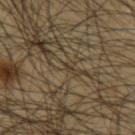| field | value |
|---|---|
| biopsy status | imaged on a skin check; not biopsied |
| lesion size | ≈1 mm |
| patient | male, roughly 45 years of age |
| imaging modality | total-body-photography crop, ~15 mm field of view |
| location | the left upper arm |